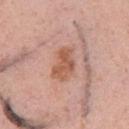Imaged during a routine full-body skin examination; the lesion was not biopsied and no histopathology is available. A 15 mm close-up extracted from a 3D total-body photography capture. The lesion is located on the left upper arm. A female patient aged approximately 35. Automated tile analysis of the lesion measured an outline eccentricity of about 0.8 (0 = round, 1 = elongated) and two-axis asymmetry of about 0.25. And it measured a mean CIELAB color near L≈57 a*≈23 b*≈31, about 10 CIELAB-L* units darker than the surrounding skin, and a normalized border contrast of about 7.5. The software also gave a border-irregularity rating of about 3/10 and internal color variation of about 3 on a 0–10 scale. It also reported a nevus-likeness score of about 65/100. Approximately 4 mm at its widest. Captured under white-light illumination.